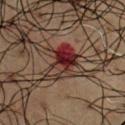{"biopsy_status": "not biopsied; imaged during a skin examination", "automated_metrics": {"eccentricity": 0.85, "shape_asymmetry": 0.45, "cielab_L": 22, "cielab_a": 20, "cielab_b": 18, "vs_skin_darker_L": 11.0, "nevus_likeness_0_100": 0, "lesion_detection_confidence_0_100": 100}, "patient": {"sex": "male", "age_approx": 60}, "image": {"source": "total-body photography crop", "field_of_view_mm": 15}, "lighting": "cross-polarized", "site": "chest", "lesion_size": {"long_diameter_mm_approx": 5.0}}